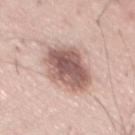Clinical impression:
Imaged during a routine full-body skin examination; the lesion was not biopsied and no histopathology is available.
Image and clinical context:
The patient is a male aged approximately 60. The lesion is located on the chest. A 15 mm close-up tile from a total-body photography series done for melanoma screening.